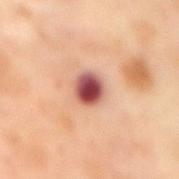workup = imaged on a skin check; not biopsied
TBP lesion metrics = a footprint of about 7 mm², a shape eccentricity near 0.4, and two-axis asymmetry of about 0.15; about 21 CIELAB-L* units darker than the surrounding skin and a normalized lesion–skin contrast near 13.5
subject = female, in their mid-50s
acquisition = ~15 mm tile from a whole-body skin photo
body site = the back
illumination = cross-polarized
lesion size = ~3 mm (longest diameter)